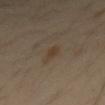notes: catalogued during a skin exam; not biopsied
TBP lesion metrics: a lesion area of about 3 mm² and a shape-asymmetry score of about 0.45 (0 = symmetric); a lesion color around L≈37 a*≈12 b*≈26 in CIELAB; an automated nevus-likeness rating near 70 out of 100 and a detector confidence of about 100 out of 100 that the crop contains a lesion
anatomic site: the mid back
lesion size: ~3 mm (longest diameter)
image source: ~15 mm tile from a whole-body skin photo
patient: male, about 50 years old
tile lighting: cross-polarized